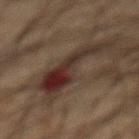Q: How was the tile lit?
A: cross-polarized illumination
Q: Patient demographics?
A: male, aged around 65
Q: Where on the body is the lesion?
A: the abdomen
Q: How was this image acquired?
A: 15 mm crop, total-body photography
Q: Automated lesion metrics?
A: a border-irregularity rating of about 7.5/10, a within-lesion color-variation index near 9/10, and peripheral color asymmetry of about 3